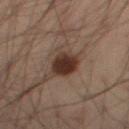On the right thigh. A male subject, in their mid- to late 50s. Automated image analysis of the tile measured an area of roughly 8 mm² and an outline eccentricity of about 0.55 (0 = round, 1 = elongated). The software also gave a border-irregularity rating of about 1.5/10 and internal color variation of about 3 on a 0–10 scale. About 3.5 mm across. This image is a 15 mm lesion crop taken from a total-body photograph.